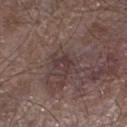workup = catalogued during a skin exam; not biopsied | lighting = white-light illumination | diameter = ≈2.5 mm | image source = 15 mm crop, total-body photography | anatomic site = the left lower leg | subject = male, aged 78 to 82.